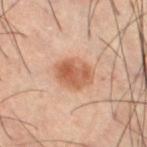| field | value |
|---|---|
| follow-up | total-body-photography surveillance lesion; no biopsy |
| acquisition | ~15 mm tile from a whole-body skin photo |
| subject | male, roughly 60 years of age |
| size | about 4.5 mm |
| location | the right thigh |
| image-analysis metrics | a footprint of about 11 mm²; a border-irregularity rating of about 1.5/10 and a color-variation rating of about 5/10; a classifier nevus-likeness of about 95/100 |
| lighting | cross-polarized |